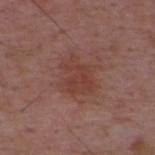Findings:
- workup · imaged on a skin check; not biopsied
- anatomic site · the upper back
- patient · male, approximately 50 years of age
- acquisition · total-body-photography crop, ~15 mm field of view
- automated metrics · a footprint of about 11 mm²; a within-lesion color-variation index near 2.5/10